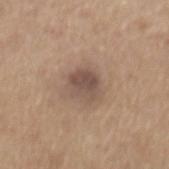Q: Was this lesion biopsied?
A: total-body-photography surveillance lesion; no biopsy
Q: Patient demographics?
A: male, in their mid-60s
Q: How was the tile lit?
A: white-light illumination
Q: Lesion location?
A: the back
Q: What did automated image analysis measure?
A: an area of roughly 7 mm², an eccentricity of roughly 0.5, and a symmetry-axis asymmetry near 0.2; roughly 10 lightness units darker than nearby skin and a lesion-to-skin contrast of about 8 (normalized; higher = more distinct); internal color variation of about 3.5 on a 0–10 scale; a nevus-likeness score of about 20/100
Q: What kind of image is this?
A: total-body-photography crop, ~15 mm field of view